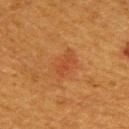Notes:
– notes · total-body-photography surveillance lesion; no biopsy
– tile lighting · cross-polarized
– imaging modality · ~15 mm crop, total-body skin-cancer survey
– lesion size · ≈2.5 mm
– anatomic site · the back
– patient · female, in their 40s